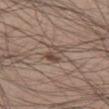biopsy status — catalogued during a skin exam; not biopsied | diameter — about 3 mm | subject — male, about 55 years old | lighting — white-light | image — 15 mm crop, total-body photography | anatomic site — the left thigh | automated lesion analysis — a mean CIELAB color near L≈47 a*≈14 b*≈23, about 9 CIELAB-L* units darker than the surrounding skin, and a normalized border contrast of about 7; a border-irregularity index near 5/10 and a color-variation rating of about 3.5/10.